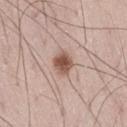Assessment: This lesion was catalogued during total-body skin photography and was not selected for biopsy. Clinical summary: Measured at roughly 3 mm in maximum diameter. Captured under white-light illumination. The lesion is on the right thigh. A 15 mm close-up tile from a total-body photography series done for melanoma screening. A male subject approximately 50 years of age.